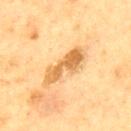notes: catalogued during a skin exam; not biopsied | TBP lesion metrics: a lesion area of about 10 mm², a shape eccentricity near 0.95, and a shape-asymmetry score of about 0.35 (0 = symmetric); a mean CIELAB color near L≈54 a*≈18 b*≈39; a nevus-likeness score of about 0/100 and lesion-presence confidence of about 100/100 | patient: male, aged approximately 75 | lesion diameter: about 6 mm | tile lighting: cross-polarized illumination | image source: ~15 mm crop, total-body skin-cancer survey | body site: the mid back.